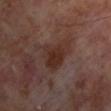Impression:
The lesion was tiled from a total-body skin photograph and was not biopsied.
Clinical summary:
On the chest. About 5 mm across. Cropped from a whole-body photographic skin survey; the tile spans about 15 mm. Captured under cross-polarized illumination. A male patient aged around 70.